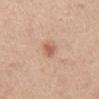workup = no biopsy performed (imaged during a skin exam) | subject = female, aged around 45 | image source = ~15 mm crop, total-body skin-cancer survey | body site = the mid back | lesion diameter = about 3 mm.